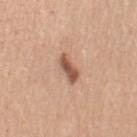A male subject, roughly 55 years of age. The lesion is on the right upper arm. Cropped from a total-body skin-imaging series; the visible field is about 15 mm.